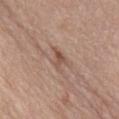Q: Is there a histopathology result?
A: imaged on a skin check; not biopsied
Q: How large is the lesion?
A: ≈3.5 mm
Q: What lighting was used for the tile?
A: white-light illumination
Q: What are the patient's age and sex?
A: female, in their mid-60s
Q: Where on the body is the lesion?
A: the mid back
Q: What is the imaging modality?
A: ~15 mm tile from a whole-body skin photo
Q: Automated lesion metrics?
A: a footprint of about 4 mm², an outline eccentricity of about 0.8 (0 = round, 1 = elongated), and a shape-asymmetry score of about 0.5 (0 = symmetric); a mean CIELAB color near L≈52 a*≈18 b*≈27 and roughly 9 lightness units darker than nearby skin; border irregularity of about 6 on a 0–10 scale, a within-lesion color-variation index near 2.5/10, and radial color variation of about 1